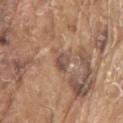body site — the right upper arm
image — ~15 mm tile from a whole-body skin photo
automated metrics — a lesion color around L≈50 a*≈18 b*≈26 in CIELAB and a lesion-to-skin contrast of about 7 (normalized; higher = more distinct); border irregularity of about 4 on a 0–10 scale, a color-variation rating of about 4.5/10, and a peripheral color-asymmetry measure near 2; an automated nevus-likeness rating near 0 out of 100 and a lesion-detection confidence of about 70/100
diameter — ~2.5 mm (longest diameter)
lighting — white-light illumination
subject — male, aged 78 to 82